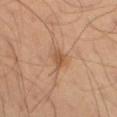The lesion was photographed on a routine skin check and not biopsied; there is no pathology result.
A 15 mm close-up extracted from a 3D total-body photography capture.
A male subject aged 48 to 52.
On the left thigh.
The tile uses cross-polarized illumination.